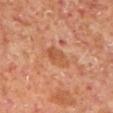Imaged during a routine full-body skin examination; the lesion was not biopsied and no histopathology is available. The recorded lesion diameter is about 3.5 mm. A male subject approximately 60 years of age. Captured under cross-polarized illumination. From the left lower leg. Automated tile analysis of the lesion measured a color-variation rating of about 3/10. The analysis additionally found a nevus-likeness score of about 0/100 and a lesion-detection confidence of about 100/100. A 15 mm close-up extracted from a 3D total-body photography capture.